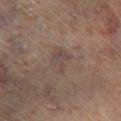notes: no biopsy performed (imaged during a skin exam)
TBP lesion metrics: a footprint of about 7 mm² and a shape eccentricity near 0.7; a detector confidence of about 95 out of 100 that the crop contains a lesion
tile lighting: cross-polarized
body site: the leg
patient: male, aged 63 to 67
size: about 3.5 mm
acquisition: 15 mm crop, total-body photography